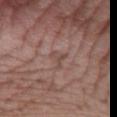The lesion is located on the arm.
A male subject, roughly 45 years of age.
Approximately 3 mm at its widest.
The total-body-photography lesion software estimated an area of roughly 3.5 mm², an eccentricity of roughly 0.75, and a symmetry-axis asymmetry near 0.6. It also reported a nevus-likeness score of about 0/100.
A region of skin cropped from a whole-body photographic capture, roughly 15 mm wide.